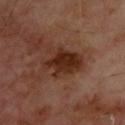Impression:
Imaged during a routine full-body skin examination; the lesion was not biopsied and no histopathology is available.
Image and clinical context:
A male patient, approximately 70 years of age. A 15 mm close-up tile from a total-body photography series done for melanoma screening. On the back. The lesion-visualizer software estimated about 11 CIELAB-L* units darker than the surrounding skin and a lesion-to-skin contrast of about 10.5 (normalized; higher = more distinct). The tile uses cross-polarized illumination.